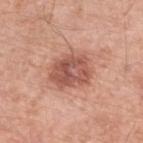{"biopsy_status": "not biopsied; imaged during a skin examination", "site": "left lower leg", "patient": {"sex": "male", "age_approx": 80}, "image": {"source": "total-body photography crop", "field_of_view_mm": 15}}